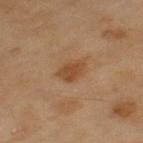Imaged during a routine full-body skin examination; the lesion was not biopsied and no histopathology is available. A 15 mm close-up extracted from a 3D total-body photography capture. The total-body-photography lesion software estimated a lesion area of about 5.5 mm², a shape eccentricity near 0.75, and two-axis asymmetry of about 0.2. The analysis additionally found border irregularity of about 2 on a 0–10 scale, internal color variation of about 2 on a 0–10 scale, and radial color variation of about 0.5. A female subject aged approximately 60. On the left thigh. This is a cross-polarized tile.